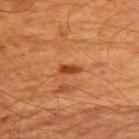Q: What lighting was used for the tile?
A: cross-polarized
Q: What did automated image analysis measure?
A: roughly 10 lightness units darker than nearby skin and a normalized lesion–skin contrast near 8.5; border irregularity of about 2.5 on a 0–10 scale, internal color variation of about 0 on a 0–10 scale, and a peripheral color-asymmetry measure near 0; an automated nevus-likeness rating near 85 out of 100
Q: How was this image acquired?
A: 15 mm crop, total-body photography
Q: What are the patient's age and sex?
A: male, roughly 60 years of age
Q: What is the anatomic site?
A: the upper back